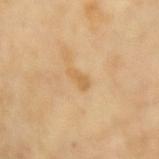| field | value |
|---|---|
| size | ~2.5 mm (longest diameter) |
| body site | the right forearm |
| image source | 15 mm crop, total-body photography |
| subject | female, in their mid- to late 70s |
| TBP lesion metrics | an area of roughly 3 mm² and a shape eccentricity near 0.9; a mean CIELAB color near L≈63 a*≈18 b*≈42, roughly 7 lightness units darker than nearby skin, and a normalized lesion–skin contrast near 5.5; a border-irregularity index near 3.5/10, a within-lesion color-variation index near 0.5/10, and a peripheral color-asymmetry measure near 0 |
| tile lighting | cross-polarized illumination |